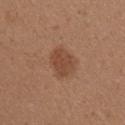workup — no biopsy performed (imaged during a skin exam)
image-analysis metrics — a footprint of about 8 mm² and a symmetry-axis asymmetry near 0.2; a nevus-likeness score of about 85/100 and a detector confidence of about 100 out of 100 that the crop contains a lesion
image — total-body-photography crop, ~15 mm field of view
body site — the right upper arm
lesion size — ~3.5 mm (longest diameter)
subject — female, in their mid-40s
tile lighting — white-light illumination